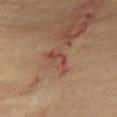Case summary:
- anatomic site — the chest
- size — ≈3.5 mm
- subject — male, roughly 70 years of age
- acquisition — ~15 mm tile from a whole-body skin photo
- automated lesion analysis — a border-irregularity rating of about 7/10 and a within-lesion color-variation index near 1/10